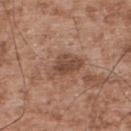imaging modality: 15 mm crop, total-body photography
subject: male, aged 53–57
size: about 4.5 mm
site: the upper back
tile lighting: white-light
automated metrics: an average lesion color of about L≈47 a*≈19 b*≈28 (CIELAB), roughly 10 lightness units darker than nearby skin, and a normalized lesion–skin contrast near 7.5; a border-irregularity rating of about 3/10, a within-lesion color-variation index near 3.5/10, and radial color variation of about 1